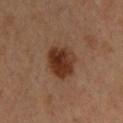Assessment:
Part of a total-body skin-imaging series; this lesion was reviewed on a skin check and was not flagged for biopsy.
Image and clinical context:
A 15 mm crop from a total-body photograph taken for skin-cancer surveillance. The lesion is on the upper back. The patient is a male about 35 years old. Captured under cross-polarized illumination. Approximately 4.5 mm at its widest.